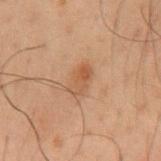Clinical impression:
The lesion was tiled from a total-body skin photograph and was not biopsied.
Clinical summary:
Cropped from a total-body skin-imaging series; the visible field is about 15 mm. Captured under cross-polarized illumination. Located on the mid back. Measured at roughly 3.5 mm in maximum diameter. The total-body-photography lesion software estimated a lesion area of about 5.5 mm², an outline eccentricity of about 0.85 (0 = round, 1 = elongated), and two-axis asymmetry of about 0.2. The software also gave a lesion color around L≈41 a*≈17 b*≈27 in CIELAB and a normalized border contrast of about 6. It also reported border irregularity of about 2 on a 0–10 scale and a color-variation rating of about 4.5/10. A male patient, approximately 50 years of age.